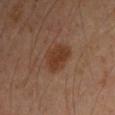Clinical impression:
No biopsy was performed on this lesion — it was imaged during a full skin examination and was not determined to be concerning.
Clinical summary:
The lesion is on the arm. A 15 mm close-up extracted from a 3D total-body photography capture. A male patient, in their mid- to late 40s.